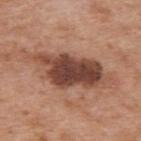biopsy status: no biopsy performed (imaged during a skin exam) | TBP lesion metrics: a shape eccentricity near 0.9 and a shape-asymmetry score of about 0.25 (0 = symmetric); a mean CIELAB color near L≈46 a*≈22 b*≈27 and a lesion–skin lightness drop of about 15; border irregularity of about 4.5 on a 0–10 scale and a color-variation rating of about 7/10 | body site: the upper back | diameter: ≈10 mm | image source: ~15 mm crop, total-body skin-cancer survey | lighting: white-light | subject: male, in their 60s.